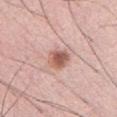This lesion was catalogued during total-body skin photography and was not selected for biopsy. The patient is a male aged around 30. Automated tile analysis of the lesion measured an average lesion color of about L≈58 a*≈23 b*≈27 (CIELAB), a lesion–skin lightness drop of about 13, and a normalized lesion–skin contrast near 9. Imaged with white-light lighting. The lesion's longest dimension is about 3 mm. A lesion tile, about 15 mm wide, cut from a 3D total-body photograph. Located on the chest.